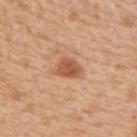Q: Was a biopsy performed?
A: total-body-photography surveillance lesion; no biopsy
Q: How was the tile lit?
A: white-light illumination
Q: Patient demographics?
A: female, aged around 55
Q: Automated lesion metrics?
A: a mean CIELAB color near L≈55 a*≈25 b*≈36, about 12 CIELAB-L* units darker than the surrounding skin, and a lesion-to-skin contrast of about 8 (normalized; higher = more distinct); a nevus-likeness score of about 95/100 and a lesion-detection confidence of about 100/100
Q: How was this image acquired?
A: 15 mm crop, total-body photography
Q: What is the anatomic site?
A: the upper back